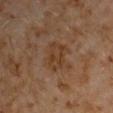The lesion was photographed on a routine skin check and not biopsied; there is no pathology result.
Approximately 5 mm at its widest.
A male subject, roughly 60 years of age.
The lesion is on the left upper arm.
Captured under cross-polarized illumination.
A roughly 15 mm field-of-view crop from a total-body skin photograph.